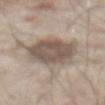follow-up = no biopsy performed (imaged during a skin exam) | imaging modality = ~15 mm tile from a whole-body skin photo | size = ~7 mm (longest diameter) | anatomic site = the abdomen | subject = male, about 80 years old.